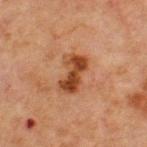Clinical impression: The lesion was photographed on a routine skin check and not biopsied; there is no pathology result. Background: From the front of the torso. The subject is a male about 65 years old. The tile uses cross-polarized illumination. Approximately 4.5 mm at its widest. A lesion tile, about 15 mm wide, cut from a 3D total-body photograph.